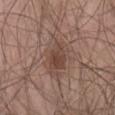Clinical summary: A male subject, in their mid- to late 70s. Cropped from a total-body skin-imaging series; the visible field is about 15 mm. The lesion is located on the abdomen. This is a white-light tile.